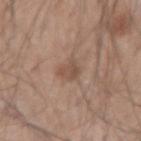Captured during whole-body skin photography for melanoma surveillance; the lesion was not biopsied.
This image is a 15 mm lesion crop taken from a total-body photograph.
A male patient in their mid- to late 40s.
On the right forearm.
Imaged with white-light lighting.
The recorded lesion diameter is about 2.5 mm.